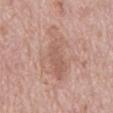  biopsy_status: not biopsied; imaged during a skin examination
  patient:
    sex: male
    age_approx: 70
  site: back
  image:
    source: total-body photography crop
    field_of_view_mm: 15
  automated_metrics:
    area_mm2_approx: 19.0
    eccentricity: 0.9
    shape_asymmetry: 0.45
    vs_skin_darker_L: 7.0
    vs_skin_contrast_norm: 5.0
    border_irregularity_0_10: 6.0
    color_variation_0_10: 4.0
    peripheral_color_asymmetry: 1.5
    nevus_likeness_0_100: 0
    lesion_detection_confidence_0_100: 100
  lesion_size:
    long_diameter_mm_approx: 8.0